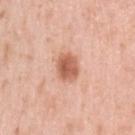  biopsy_status: not biopsied; imaged during a skin examination
  site: left upper arm
  lighting: white-light
  patient:
    sex: female
    age_approx: 40
  automated_metrics:
    area_mm2_approx: 7.5
    shape_asymmetry: 0.1
    cielab_L: 60
    cielab_a: 26
    cielab_b: 32
    vs_skin_darker_L: 14.0
    vs_skin_contrast_norm: 9.0
    border_irregularity_0_10: 1.5
    color_variation_0_10: 4.0
    peripheral_color_asymmetry: 1.0
    nevus_likeness_0_100: 95
  image:
    source: total-body photography crop
    field_of_view_mm: 15